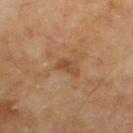The lesion was photographed on a routine skin check and not biopsied; there is no pathology result.
Located on the upper back.
Cropped from a total-body skin-imaging series; the visible field is about 15 mm.
The subject is a male approximately 55 years of age.
The lesion's longest dimension is about 2.5 mm.
The tile uses cross-polarized illumination.